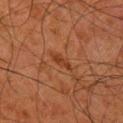follow-up: total-body-photography surveillance lesion; no biopsy | patient: male, about 80 years old | acquisition: ~15 mm crop, total-body skin-cancer survey | illumination: cross-polarized illumination | TBP lesion metrics: an eccentricity of roughly 0.9 and a shape-asymmetry score of about 0.35 (0 = symmetric); a mean CIELAB color near L≈30 a*≈21 b*≈29; border irregularity of about 3.5 on a 0–10 scale and a color-variation rating of about 0/10; a lesion-detection confidence of about 80/100 | body site: the right lower leg | lesion size: ≈2.5 mm.